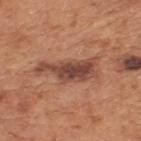Impression:
This lesion was catalogued during total-body skin photography and was not selected for biopsy.
Acquisition and patient details:
Captured under white-light illumination. On the upper back. A 15 mm crop from a total-body photograph taken for skin-cancer surveillance. Automated image analysis of the tile measured a classifier nevus-likeness of about 20/100 and a lesion-detection confidence of about 100/100. The patient is a male in their mid-60s. The recorded lesion diameter is about 6.5 mm.